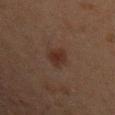No biopsy was performed on this lesion — it was imaged during a full skin examination and was not determined to be concerning. A 15 mm crop from a total-body photograph taken for skin-cancer surveillance. A male patient aged 43 to 47. From the left upper arm. Captured under cross-polarized illumination. The recorded lesion diameter is about 3 mm. The lesion-visualizer software estimated internal color variation of about 3 on a 0–10 scale and peripheral color asymmetry of about 1.